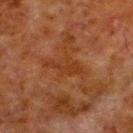Case summary:
• follow-up — total-body-photography surveillance lesion; no biopsy
• size — ≈5 mm
• tile lighting — cross-polarized illumination
• patient — male, about 80 years old
• image — 15 mm crop, total-body photography
• site — the left lower leg